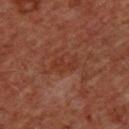follow-up: imaged on a skin check; not biopsied | image-analysis metrics: a mean CIELAB color near L≈31 a*≈24 b*≈27 and roughly 5 lightness units darker than nearby skin; an automated nevus-likeness rating near 0 out of 100 and a lesion-detection confidence of about 100/100 | anatomic site: the chest | lesion size: ≈3 mm | subject: male, aged around 60 | acquisition: ~15 mm crop, total-body skin-cancer survey.